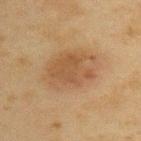Imaged during a routine full-body skin examination; the lesion was not biopsied and no histopathology is available. An algorithmic analysis of the crop reported an area of roughly 21 mm², an eccentricity of roughly 0.75, and a shape-asymmetry score of about 0.1 (0 = symmetric). Approximately 6.5 mm at its widest. Imaged with cross-polarized lighting. A male patient about 45 years old. From the upper back. Cropped from a whole-body photographic skin survey; the tile spans about 15 mm.